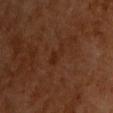Case summary:
• biopsy status: no biopsy performed (imaged during a skin exam)
• image source: total-body-photography crop, ~15 mm field of view
• body site: the chest
• patient: male, approximately 65 years of age